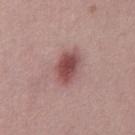Imaged during a routine full-body skin examination; the lesion was not biopsied and no histopathology is available.
A male patient aged approximately 30.
A close-up tile cropped from a whole-body skin photograph, about 15 mm across.
The lesion-visualizer software estimated a lesion area of about 8 mm², an eccentricity of roughly 0.75, and a symmetry-axis asymmetry near 0.15. It also reported a mean CIELAB color near L≈48 a*≈24 b*≈21, a lesion–skin lightness drop of about 13, and a lesion-to-skin contrast of about 9.5 (normalized; higher = more distinct). It also reported a border-irregularity index near 2/10, a within-lesion color-variation index near 4/10, and peripheral color asymmetry of about 1. It also reported an automated nevus-likeness rating near 90 out of 100 and lesion-presence confidence of about 100/100.